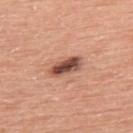Q: Was this lesion biopsied?
A: no biopsy performed (imaged during a skin exam)
Q: What are the patient's age and sex?
A: male, aged around 60
Q: What kind of image is this?
A: total-body-photography crop, ~15 mm field of view
Q: Lesion location?
A: the upper back